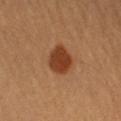Notes:
- biopsy status · total-body-photography surveillance lesion; no biopsy
- lighting · cross-polarized
- automated metrics · border irregularity of about 1.5 on a 0–10 scale, a color-variation rating of about 2/10, and radial color variation of about 0.5
- body site · the left upper arm
- image · ~15 mm crop, total-body skin-cancer survey
- patient · male, about 60 years old